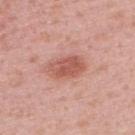Recorded during total-body skin imaging; not selected for excision or biopsy. The lesion-visualizer software estimated a classifier nevus-likeness of about 95/100 and lesion-presence confidence of about 100/100. Cropped from a whole-body photographic skin survey; the tile spans about 15 mm. From the upper back. About 4 mm across. The subject is a female aged 38–42. Imaged with white-light lighting.